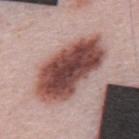• workup: no biopsy performed (imaged during a skin exam)
• body site: the upper back
• lesion diameter: about 9.5 mm
• lighting: white-light illumination
• acquisition: ~15 mm crop, total-body skin-cancer survey
• image-analysis metrics: a lesion area of about 36 mm² and a shape-asymmetry score of about 0.15 (0 = symmetric)
• subject: male, roughly 50 years of age